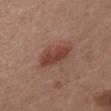Q: Was a biopsy performed?
A: catalogued during a skin exam; not biopsied
Q: How was this image acquired?
A: total-body-photography crop, ~15 mm field of view
Q: Lesion location?
A: the chest
Q: How was the tile lit?
A: white-light
Q: What are the patient's age and sex?
A: female, aged 28–32